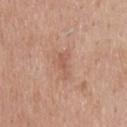Assessment:
The lesion was tiled from a total-body skin photograph and was not biopsied.
Clinical summary:
From the upper back. A male subject, aged 43–47. The tile uses white-light illumination. A close-up tile cropped from a whole-body skin photograph, about 15 mm across.